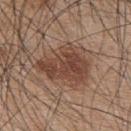Context: A 15 mm close-up extracted from a 3D total-body photography capture. A male subject, aged 43 to 47. An algorithmic analysis of the crop reported a lesion area of about 17 mm², an eccentricity of roughly 0.75, and two-axis asymmetry of about 0.45. The analysis additionally found a lesion–skin lightness drop of about 11. And it measured lesion-presence confidence of about 100/100. The lesion is located on the upper back. Longest diameter approximately 6 mm.